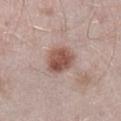Image and clinical context: A male patient, aged 38 to 42. Longest diameter approximately 3.5 mm. This image is a 15 mm lesion crop taken from a total-body photograph. The tile uses white-light illumination. The lesion is on the right lower leg.